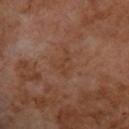A male subject about 70 years old.
A region of skin cropped from a whole-body photographic capture, roughly 15 mm wide.
The lesion is on the upper back.
The lesion-visualizer software estimated a lesion area of about 2.5 mm², an outline eccentricity of about 0.9 (0 = round, 1 = elongated), and a symmetry-axis asymmetry near 0.55. It also reported a border-irregularity rating of about 9/10, a color-variation rating of about 0/10, and peripheral color asymmetry of about 0. And it measured lesion-presence confidence of about 100/100.
This is a cross-polarized tile.
Measured at roughly 3 mm in maximum diameter.